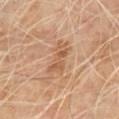The lesion was photographed on a routine skin check and not biopsied; there is no pathology result. An algorithmic analysis of the crop reported a border-irregularity rating of about 6/10, internal color variation of about 2 on a 0–10 scale, and a peripheral color-asymmetry measure near 0. From the front of the torso. The lesion's longest dimension is about 4 mm. This image is a 15 mm lesion crop taken from a total-body photograph. A male subject aged 68–72. The tile uses cross-polarized illumination.